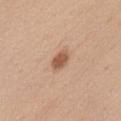biopsy status=total-body-photography surveillance lesion; no biopsy | diameter=~2.5 mm (longest diameter) | location=the front of the torso | automated metrics=a lesion area of about 4 mm² and a shape eccentricity near 0.8; a border-irregularity index near 2/10, a color-variation rating of about 1.5/10, and peripheral color asymmetry of about 0.5 | patient=male, in their 40s | lighting=white-light illumination | image=~15 mm tile from a whole-body skin photo.